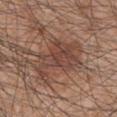No biopsy was performed on this lesion — it was imaged during a full skin examination and was not determined to be concerning. The subject is a male approximately 45 years of age. The tile uses white-light illumination. Longest diameter approximately 6.5 mm. A roughly 15 mm field-of-view crop from a total-body skin photograph. The lesion is on the left upper arm.